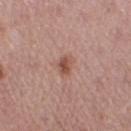Imaged during a routine full-body skin examination; the lesion was not biopsied and no histopathology is available.